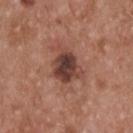biopsy_status: not biopsied; imaged during a skin examination
patient:
  sex: male
  age_approx: 55
site: upper back
lighting: white-light
automated_metrics:
  cielab_L: 41
  cielab_a: 22
  cielab_b: 24
  vs_skin_darker_L: 13.0
  vs_skin_contrast_norm: 10.5
  border_irregularity_0_10: 3.5
  color_variation_0_10: 6.0
image:
  source: total-body photography crop
  field_of_view_mm: 15
lesion_size:
  long_diameter_mm_approx: 5.0